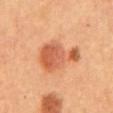Q: Was a biopsy performed?
A: total-body-photography surveillance lesion; no biopsy
Q: Patient demographics?
A: female
Q: What did automated image analysis measure?
A: a footprint of about 17 mm², a shape eccentricity near 0.65, and two-axis asymmetry of about 0.4; a within-lesion color-variation index near 5.5/10 and peripheral color asymmetry of about 1.5; a nevus-likeness score of about 100/100
Q: How large is the lesion?
A: about 5.5 mm
Q: What lighting was used for the tile?
A: cross-polarized illumination
Q: What is the imaging modality?
A: 15 mm crop, total-body photography
Q: What is the anatomic site?
A: the mid back